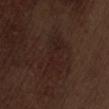biopsy status: catalogued during a skin exam; not biopsied | location: the lower back | subject: male, aged 68–72 | illumination: white-light | TBP lesion metrics: a footprint of about 9 mm², a shape eccentricity near 0.95, and a shape-asymmetry score of about 0.45 (0 = symmetric); roughly 4 lightness units darker than nearby skin and a normalized lesion–skin contrast near 6; a border-irregularity rating of about 6.5/10 and a color-variation rating of about 3/10; a classifier nevus-likeness of about 5/100 | image: ~15 mm tile from a whole-body skin photo | lesion size: ≈6 mm.